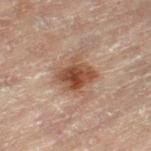follow-up: imaged on a skin check; not biopsied | patient: female, approximately 80 years of age | body site: the leg | lighting: cross-polarized | imaging modality: 15 mm crop, total-body photography.